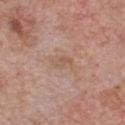This lesion was catalogued during total-body skin photography and was not selected for biopsy.